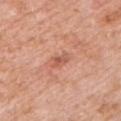Assessment:
This lesion was catalogued during total-body skin photography and was not selected for biopsy.
Background:
On the left upper arm. A male subject aged around 60. About 2.5 mm across. The total-body-photography lesion software estimated a footprint of about 3 mm², an eccentricity of roughly 0.85, and two-axis asymmetry of about 0.25. It also reported a border-irregularity index near 2.5/10, a color-variation rating of about 2/10, and peripheral color asymmetry of about 0.5. A 15 mm crop from a total-body photograph taken for skin-cancer surveillance.